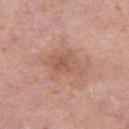Q: Was a biopsy performed?
A: total-body-photography surveillance lesion; no biopsy
Q: Patient demographics?
A: female, aged approximately 40
Q: What lighting was used for the tile?
A: white-light illumination
Q: What is the anatomic site?
A: the right thigh
Q: What kind of image is this?
A: total-body-photography crop, ~15 mm field of view
Q: What is the lesion's diameter?
A: ~5 mm (longest diameter)